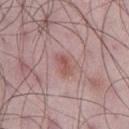| feature | finding |
|---|---|
| biopsy status | no biopsy performed (imaged during a skin exam) |
| illumination | white-light |
| imaging modality | ~15 mm crop, total-body skin-cancer survey |
| subject | male, aged around 50 |
| lesion diameter | ~3 mm (longest diameter) |
| body site | the left thigh |